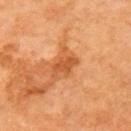Case summary:
- biopsy status · imaged on a skin check; not biopsied
- anatomic site · the arm
- subject · female, aged 63–67
- image · ~15 mm crop, total-body skin-cancer survey
- automated metrics · an area of roughly 4.5 mm², an eccentricity of roughly 0.75, and a symmetry-axis asymmetry near 0.2; a lesion color around L≈53 a*≈30 b*≈43 in CIELAB, a lesion–skin lightness drop of about 10, and a lesion-to-skin contrast of about 7 (normalized; higher = more distinct); a color-variation rating of about 2.5/10 and radial color variation of about 1; an automated nevus-likeness rating near 0 out of 100 and a lesion-detection confidence of about 100/100
- size · ≈3 mm